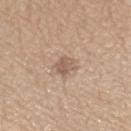Part of a total-body skin-imaging series; this lesion was reviewed on a skin check and was not flagged for biopsy.
Located on the left forearm.
Cropped from a whole-body photographic skin survey; the tile spans about 15 mm.
The subject is a male approximately 65 years of age.